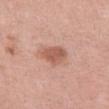Impression: Captured during whole-body skin photography for melanoma surveillance; the lesion was not biopsied. Image and clinical context: A female subject, roughly 40 years of age. Cropped from a whole-body photographic skin survey; the tile spans about 15 mm. The total-body-photography lesion software estimated a footprint of about 7.5 mm², a shape eccentricity near 0.75, and a shape-asymmetry score of about 0.25 (0 = symmetric). The software also gave an average lesion color of about L≈57 a*≈24 b*≈29 (CIELAB), a lesion–skin lightness drop of about 11, and a lesion-to-skin contrast of about 7 (normalized; higher = more distinct). And it measured border irregularity of about 2 on a 0–10 scale, internal color variation of about 2 on a 0–10 scale, and peripheral color asymmetry of about 1. Captured under white-light illumination. Measured at roughly 4 mm in maximum diameter. The lesion is located on the left thigh.